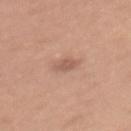Assessment:
The lesion was photographed on a routine skin check and not biopsied; there is no pathology result.
Image and clinical context:
The total-body-photography lesion software estimated a mean CIELAB color near L≈57 a*≈21 b*≈27, a lesion–skin lightness drop of about 9, and a lesion-to-skin contrast of about 6 (normalized; higher = more distinct). The analysis additionally found border irregularity of about 1.5 on a 0–10 scale, a color-variation rating of about 1/10, and peripheral color asymmetry of about 0.5. This is a white-light tile. About 2.5 mm across. Cropped from a total-body skin-imaging series; the visible field is about 15 mm. On the mid back. A female subject, in their 50s.